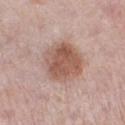Clinical impression: The lesion was tiled from a total-body skin photograph and was not biopsied. Image and clinical context: This image is a 15 mm lesion crop taken from a total-body photograph. Approximately 5 mm at its widest. Automated tile analysis of the lesion measured a mean CIELAB color near L≈55 a*≈20 b*≈27, a lesion–skin lightness drop of about 12, and a lesion-to-skin contrast of about 8.5 (normalized; higher = more distinct). And it measured border irregularity of about 1.5 on a 0–10 scale, internal color variation of about 4.5 on a 0–10 scale, and peripheral color asymmetry of about 1.5. The analysis additionally found an automated nevus-likeness rating near 50 out of 100 and a lesion-detection confidence of about 100/100. This is a white-light tile. Located on the left lower leg. A female subject roughly 40 years of age.